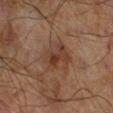The lesion was tiled from a total-body skin photograph and was not biopsied. A 15 mm crop from a total-body photograph taken for skin-cancer surveillance. Located on the right lower leg. Captured under cross-polarized illumination. The lesion-visualizer software estimated a classifier nevus-likeness of about 5/100 and lesion-presence confidence of about 100/100. A male patient aged around 70. Longest diameter approximately 4 mm.